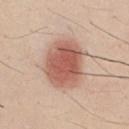notes: total-body-photography surveillance lesion; no biopsy
image: 15 mm crop, total-body photography
subject: male, aged 33 to 37
site: the front of the torso
lesion diameter: about 6 mm